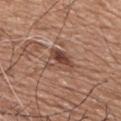The lesion was photographed on a routine skin check and not biopsied; there is no pathology result. A 15 mm close-up extracted from a 3D total-body photography capture. A male subject in their mid-70s. An algorithmic analysis of the crop reported a lesion area of about 7.5 mm², an outline eccentricity of about 0.8 (0 = round, 1 = elongated), and two-axis asymmetry of about 0.35. And it measured an average lesion color of about L≈46 a*≈20 b*≈27 (CIELAB), a lesion–skin lightness drop of about 10, and a normalized border contrast of about 8. And it measured border irregularity of about 4 on a 0–10 scale and radial color variation of about 3. It also reported a classifier nevus-likeness of about 50/100 and lesion-presence confidence of about 95/100. Captured under white-light illumination. Measured at roughly 4 mm in maximum diameter. From the head or neck.